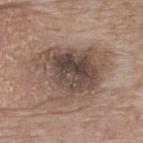follow-up = catalogued during a skin exam; not biopsied | automated metrics = an area of roughly 34 mm², a shape eccentricity near 0.6, and a symmetry-axis asymmetry near 0.4; a lesion-detection confidence of about 100/100 | lighting = white-light | patient = female, aged around 75 | location = the upper back | size = ≈8 mm | image = total-body-photography crop, ~15 mm field of view.